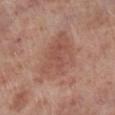notes = catalogued during a skin exam; not biopsied.